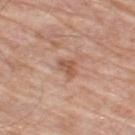workup — total-body-photography surveillance lesion; no biopsy
image — total-body-photography crop, ~15 mm field of view
lesion size — ~2.5 mm (longest diameter)
subject — male, aged 78 to 82
TBP lesion metrics — an area of roughly 3.5 mm² and an outline eccentricity of about 0.8 (0 = round, 1 = elongated); a lesion color around L≈55 a*≈22 b*≈30 in CIELAB, a lesion–skin lightness drop of about 10, and a normalized lesion–skin contrast near 7; an automated nevus-likeness rating near 0 out of 100 and a detector confidence of about 100 out of 100 that the crop contains a lesion
tile lighting — white-light illumination
anatomic site — the leg